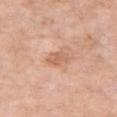This lesion was catalogued during total-body skin photography and was not selected for biopsy.
The lesion is on the chest.
About 3 mm across.
Cropped from a total-body skin-imaging series; the visible field is about 15 mm.
A female patient, in their mid- to late 70s.
Captured under white-light illumination.